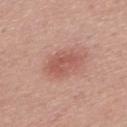{
  "site": "mid back",
  "image": {
    "source": "total-body photography crop",
    "field_of_view_mm": 15
  },
  "patient": {
    "sex": "male",
    "age_approx": 65
  }
}